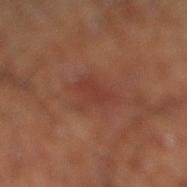Notes:
* notes · catalogued during a skin exam; not biopsied
* subject · male, roughly 60 years of age
* automated metrics · an outline eccentricity of about 0.75 (0 = round, 1 = elongated) and a symmetry-axis asymmetry near 0.4; a border-irregularity index near 4.5/10, a within-lesion color-variation index near 1.5/10, and peripheral color asymmetry of about 0.5
* illumination · cross-polarized illumination
* lesion size · ≈4 mm
* image source · ~15 mm crop, total-body skin-cancer survey
* body site · the leg